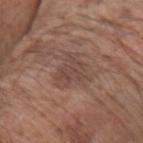Imaged during a routine full-body skin examination; the lesion was not biopsied and no histopathology is available. The subject is a male aged 68 to 72. A close-up tile cropped from a whole-body skin photograph, about 15 mm across. From the arm.